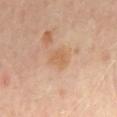This lesion was catalogued during total-body skin photography and was not selected for biopsy.